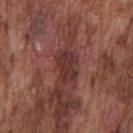Notes:
* notes · no biopsy performed (imaged during a skin exam)
* body site · the chest
* automated metrics · a border-irregularity index near 3/10, a color-variation rating of about 2.5/10, and a peripheral color-asymmetry measure near 1; a nevus-likeness score of about 0/100 and a detector confidence of about 80 out of 100 that the crop contains a lesion
* lesion diameter · about 3.5 mm
* image source · total-body-photography crop, ~15 mm field of view
* patient · male, in their mid- to late 70s